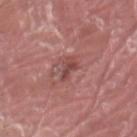• biopsy status · catalogued during a skin exam; not biopsied
• body site · the left thigh
• acquisition · 15 mm crop, total-body photography
• patient · male, in their 40s